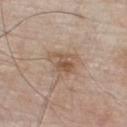Assessment: No biopsy was performed on this lesion — it was imaged during a full skin examination and was not determined to be concerning. Background: From the chest. Longest diameter approximately 3.5 mm. The total-body-photography lesion software estimated a footprint of about 8 mm², a shape eccentricity near 0.55, and two-axis asymmetry of about 0.3. It also reported a classifier nevus-likeness of about 35/100 and a detector confidence of about 100 out of 100 that the crop contains a lesion. The patient is a male approximately 80 years of age. Captured under white-light illumination. A roughly 15 mm field-of-view crop from a total-body skin photograph.